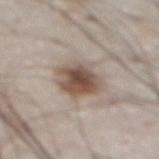Q: Was this lesion biopsied?
A: total-body-photography surveillance lesion; no biopsy
Q: What did automated image analysis measure?
A: a footprint of about 13 mm², an eccentricity of roughly 0.7, and a shape-asymmetry score of about 0.15 (0 = symmetric); border irregularity of about 2 on a 0–10 scale, internal color variation of about 6 on a 0–10 scale, and a peripheral color-asymmetry measure near 1.5
Q: What is the anatomic site?
A: the abdomen
Q: How was this image acquired?
A: ~15 mm tile from a whole-body skin photo
Q: Who is the patient?
A: male, about 80 years old